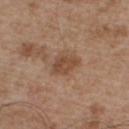Recorded during total-body skin imaging; not selected for excision or biopsy.
The lesion is on the chest.
A male subject aged 53 to 57.
Captured under white-light illumination.
The lesion's longest dimension is about 3 mm.
A 15 mm crop from a total-body photograph taken for skin-cancer surveillance.